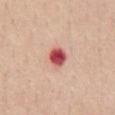  biopsy_status: not biopsied; imaged during a skin examination
  lesion_size:
    long_diameter_mm_approx: 2.5
  image:
    source: total-body photography crop
    field_of_view_mm: 15
  patient:
    sex: female
    age_approx: 65
  lighting: white-light
  site: abdomen